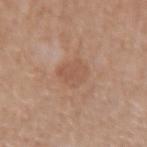workup = catalogued during a skin exam; not biopsied | anatomic site = the right forearm | imaging modality = ~15 mm tile from a whole-body skin photo | patient = female, in their mid- to late 50s.